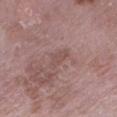Part of a total-body skin-imaging series; this lesion was reviewed on a skin check and was not flagged for biopsy.
The patient is a female aged approximately 70.
The lesion's longest dimension is about 3.5 mm.
The lesion is on the leg.
This is a white-light tile.
A region of skin cropped from a whole-body photographic capture, roughly 15 mm wide.